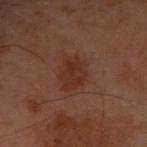Findings:
– workup — imaged on a skin check; not biopsied
– site — the front of the torso
– acquisition — total-body-photography crop, ~15 mm field of view
– subject — female, aged approximately 60